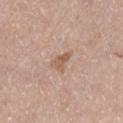Case summary:
* biopsy status · total-body-photography surveillance lesion; no biopsy
* automated metrics · a within-lesion color-variation index near 2.5/10
* patient · female, roughly 60 years of age
* acquisition · total-body-photography crop, ~15 mm field of view
* lesion diameter · ≈2.5 mm
* site · the right lower leg